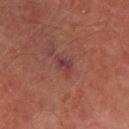<record>
<biopsy_status>not biopsied; imaged during a skin examination</biopsy_status>
<image>
  <source>total-body photography crop</source>
  <field_of_view_mm>15</field_of_view_mm>
</image>
<lighting>cross-polarized</lighting>
<lesion_size>
  <long_diameter_mm_approx>2.5</long_diameter_mm_approx>
</lesion_size>
<site>left thigh</site>
<patient>
  <sex>male</sex>
  <age_approx>75</age_approx>
</patient>
<automated_metrics>
  <nevus_likeness_0_100>0</nevus_likeness_0_100>
  <lesion_detection_confidence_0_100>95</lesion_detection_confidence_0_100>
</automated_metrics>
</record>